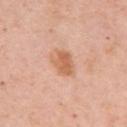Part of a total-body skin-imaging series; this lesion was reviewed on a skin check and was not flagged for biopsy. A region of skin cropped from a whole-body photographic capture, roughly 15 mm wide. The lesion is on the left upper arm. The lesion-visualizer software estimated a nevus-likeness score of about 45/100 and a lesion-detection confidence of about 100/100. A female subject, approximately 65 years of age. The tile uses white-light illumination. About 3.5 mm across.